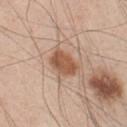Q: Lesion location?
A: the chest
Q: How was this image acquired?
A: total-body-photography crop, ~15 mm field of view
Q: How large is the lesion?
A: about 3.5 mm
Q: What are the patient's age and sex?
A: male, in their mid- to late 40s
Q: What lighting was used for the tile?
A: white-light